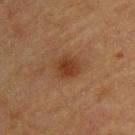<case>
<biopsy_status>not biopsied; imaged during a skin examination</biopsy_status>
<patient>
  <sex>male</sex>
  <age_approx>60</age_approx>
</patient>
<site>upper back</site>
<image>
  <source>total-body photography crop</source>
  <field_of_view_mm>15</field_of_view_mm>
</image>
<lighting>cross-polarized</lighting>
</case>